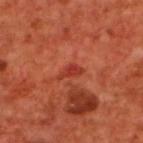This lesion was catalogued during total-body skin photography and was not selected for biopsy. An algorithmic analysis of the crop reported a lesion area of about 3.5 mm² and a shape-asymmetry score of about 0.4 (0 = symmetric). It also reported border irregularity of about 4 on a 0–10 scale, internal color variation of about 1.5 on a 0–10 scale, and radial color variation of about 0.5. And it measured a classifier nevus-likeness of about 0/100 and lesion-presence confidence of about 95/100. Longest diameter approximately 3 mm. Located on the back. The tile uses cross-polarized illumination. A 15 mm close-up extracted from a 3D total-body photography capture. A male subject aged 68 to 72.